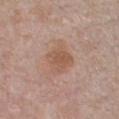biopsy status=total-body-photography surveillance lesion; no biopsy
illumination=white-light
size=≈5 mm
anatomic site=the chest
subject=male, roughly 75 years of age
imaging modality=~15 mm tile from a whole-body skin photo